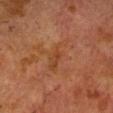Imaged during a routine full-body skin examination; the lesion was not biopsied and no histopathology is available. The lesion is on the head or neck. Longest diameter approximately 5 mm. This image is a 15 mm lesion crop taken from a total-body photograph. A male subject, about 75 years old.